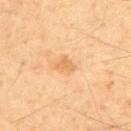Assessment: The lesion was tiled from a total-body skin photograph and was not biopsied. Background: The subject is a male aged approximately 55. Imaged with cross-polarized lighting. Automated tile analysis of the lesion measured an area of roughly 3.5 mm², an outline eccentricity of about 0.75 (0 = round, 1 = elongated), and a shape-asymmetry score of about 0.4 (0 = symmetric). The analysis additionally found a mean CIELAB color near L≈68 a*≈21 b*≈43, a lesion–skin lightness drop of about 8, and a normalized border contrast of about 5.5. A 15 mm close-up extracted from a 3D total-body photography capture. The recorded lesion diameter is about 3 mm. Located on the upper back.